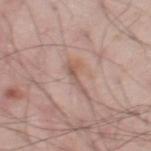workup = no biopsy performed (imaged during a skin exam)
subject = male, aged 48 to 52
automated metrics = a lesion area of about 3.5 mm² and two-axis asymmetry of about 0.55; a detector confidence of about 60 out of 100 that the crop contains a lesion
anatomic site = the right thigh
acquisition = 15 mm crop, total-body photography
diameter = ≈4 mm
tile lighting = white-light